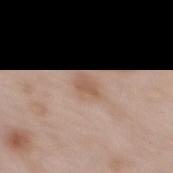follow-up: no biopsy performed (imaged during a skin exam); imaging modality: 15 mm crop, total-body photography; lesion size: about 2.5 mm; site: the back; patient: female, aged around 65.